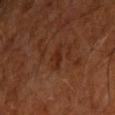Notes:
- biopsy status · catalogued during a skin exam; not biopsied